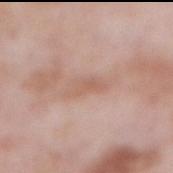follow-up = total-body-photography surveillance lesion; no biopsy
illumination = white-light
subject = male, aged 53 to 57
lesion diameter = ~4 mm (longest diameter)
anatomic site = the abdomen
imaging modality = 15 mm crop, total-body photography